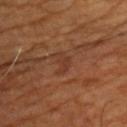<tbp_lesion>
  <biopsy_status>not biopsied; imaged during a skin examination</biopsy_status>
  <patient>
    <sex>male</sex>
    <age_approx>65</age_approx>
  </patient>
  <image>
    <source>total-body photography crop</source>
    <field_of_view_mm>15</field_of_view_mm>
  </image>
  <site>chest</site>
</tbp_lesion>